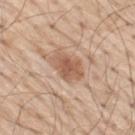Assessment:
The lesion was tiled from a total-body skin photograph and was not biopsied.
Context:
Located on the mid back. A 15 mm close-up extracted from a 3D total-body photography capture. A male subject, roughly 70 years of age.